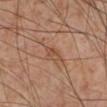{"biopsy_status": "not biopsied; imaged during a skin examination", "patient": {"sex": "male", "age_approx": 55}, "site": "right lower leg", "lesion_size": {"long_diameter_mm_approx": 3.0}, "image": {"source": "total-body photography crop", "field_of_view_mm": 15}, "automated_metrics": {"shape_asymmetry": 0.3, "vs_skin_darker_L": 7.0, "vs_skin_contrast_norm": 5.5, "nevus_likeness_0_100": 10, "lesion_detection_confidence_0_100": 95}}